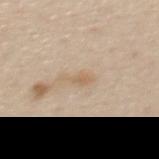No biopsy was performed on this lesion — it was imaged during a full skin examination and was not determined to be concerning. From the upper back. A female patient, approximately 45 years of age. Cropped from a total-body skin-imaging series; the visible field is about 15 mm. The lesion's longest dimension is about 3 mm.